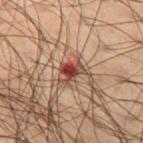Imaged during a routine full-body skin examination; the lesion was not biopsied and no histopathology is available.
The lesion is on the left thigh.
The lesion-visualizer software estimated a lesion area of about 4.5 mm², a shape eccentricity near 0.65, and a symmetry-axis asymmetry near 0.45. The analysis additionally found a border-irregularity index near 4.5/10 and a within-lesion color-variation index near 8.5/10. And it measured a classifier nevus-likeness of about 0/100 and a detector confidence of about 100 out of 100 that the crop contains a lesion.
A region of skin cropped from a whole-body photographic capture, roughly 15 mm wide.
The patient is a male about 50 years old.